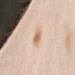Captured during whole-body skin photography for melanoma surveillance; the lesion was not biopsied. Located on the left arm. Approximately 3 mm at its widest. This is a cross-polarized tile. A female patient, aged 38 to 42. A 15 mm close-up extracted from a 3D total-body photography capture. Automated image analysis of the tile measured a mean CIELAB color near L≈68 a*≈19 b*≈33, a lesion–skin lightness drop of about 11, and a lesion-to-skin contrast of about 7.5 (normalized; higher = more distinct). And it measured a border-irregularity rating of about 2.5/10, a within-lesion color-variation index near 1.5/10, and radial color variation of about 0.5.